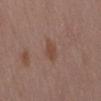<tbp_lesion>
  <biopsy_status>not biopsied; imaged during a skin examination</biopsy_status>
  <site>abdomen</site>
  <patient>
    <sex>female</sex>
    <age_approx>70</age_approx>
  </patient>
  <lesion_size>
    <long_diameter_mm_approx>3.5</long_diameter_mm_approx>
  </lesion_size>
  <image>
    <source>total-body photography crop</source>
    <field_of_view_mm>15</field_of_view_mm>
  </image>
  <lighting>white-light</lighting>
</tbp_lesion>